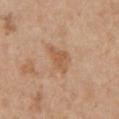notes: imaged on a skin check; not biopsied | anatomic site: the chest | diameter: ≈3.5 mm | acquisition: ~15 mm crop, total-body skin-cancer survey | patient: male, aged around 65 | lighting: white-light | automated lesion analysis: an area of roughly 6.5 mm², a shape eccentricity near 0.7, and two-axis asymmetry of about 0.35; border irregularity of about 3.5 on a 0–10 scale, internal color variation of about 2 on a 0–10 scale, and peripheral color asymmetry of about 0.5; an automated nevus-likeness rating near 0 out of 100 and a detector confidence of about 100 out of 100 that the crop contains a lesion.